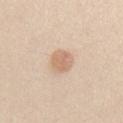Assessment: Captured during whole-body skin photography for melanoma surveillance; the lesion was not biopsied. Background: The patient is a male roughly 25 years of age. A 15 mm crop from a total-body photograph taken for skin-cancer surveillance. Located on the chest.